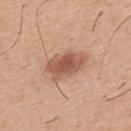{"biopsy_status": "not biopsied; imaged during a skin examination", "lighting": "white-light", "site": "upper back", "lesion_size": {"long_diameter_mm_approx": 5.0}, "patient": {"sex": "male", "age_approx": 40}, "image": {"source": "total-body photography crop", "field_of_view_mm": 15}}